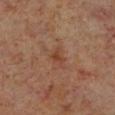Background: On the leg. A male patient, approximately 60 years of age. The tile uses cross-polarized illumination. The lesion-visualizer software estimated an area of roughly 3.5 mm² and an outline eccentricity of about 0.75 (0 = round, 1 = elongated). And it measured a detector confidence of about 100 out of 100 that the crop contains a lesion. About 2.5 mm across. A roughly 15 mm field-of-view crop from a total-body skin photograph.